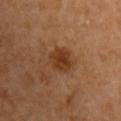Assessment:
Imaged during a routine full-body skin examination; the lesion was not biopsied and no histopathology is available.